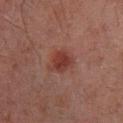A male subject in their mid-60s. The lesion-visualizer software estimated a footprint of about 7 mm², an eccentricity of roughly 0.6, and two-axis asymmetry of about 0.25. And it measured border irregularity of about 2.5 on a 0–10 scale, internal color variation of about 2.5 on a 0–10 scale, and radial color variation of about 1. The software also gave a classifier nevus-likeness of about 95/100 and lesion-presence confidence of about 100/100. Cropped from a total-body skin-imaging series; the visible field is about 15 mm. The lesion's longest dimension is about 3.5 mm. This is a cross-polarized tile. Located on the leg.